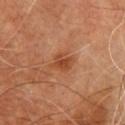follow-up: catalogued during a skin exam; not biopsied
site: the chest
illumination: cross-polarized illumination
image source: ~15 mm crop, total-body skin-cancer survey
patient: male, in their mid-50s